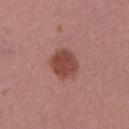Cropped from a whole-body photographic skin survey; the tile spans about 15 mm.
An algorithmic analysis of the crop reported an outline eccentricity of about 0.4 (0 = round, 1 = elongated) and a shape-asymmetry score of about 0.1 (0 = symmetric). The software also gave a mean CIELAB color near L≈46 a*≈26 b*≈26, roughly 12 lightness units darker than nearby skin, and a normalized border contrast of about 9. The software also gave a detector confidence of about 100 out of 100 that the crop contains a lesion.
Measured at roughly 3.5 mm in maximum diameter.
The lesion is located on the leg.
The subject is a female about 15 years old.